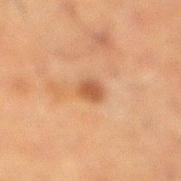workup: no biopsy performed (imaged during a skin exam) | location: the right lower leg | automated lesion analysis: a lesion area of about 4 mm², an outline eccentricity of about 0.65 (0 = round, 1 = elongated), and a symmetry-axis asymmetry near 0.25; border irregularity of about 2 on a 0–10 scale, internal color variation of about 2.5 on a 0–10 scale, and radial color variation of about 1; a lesion-detection confidence of about 100/100 | lesion diameter: ~2.5 mm (longest diameter) | subject: male, aged approximately 60 | acquisition: total-body-photography crop, ~15 mm field of view | tile lighting: cross-polarized illumination.